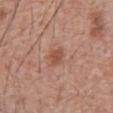patient = male, in their 60s | diameter = about 2.5 mm | anatomic site = the abdomen | imaging modality = 15 mm crop, total-body photography.